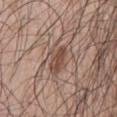notes: no biopsy performed (imaged during a skin exam)
patient: male, aged approximately 55
body site: the chest
tile lighting: white-light illumination
image-analysis metrics: an eccentricity of roughly 0.85 and a shape-asymmetry score of about 0.4 (0 = symmetric); a within-lesion color-variation index near 4/10; a lesion-detection confidence of about 90/100
size: about 5 mm
acquisition: 15 mm crop, total-body photography